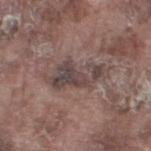illumination: white-light illumination | size: about 6.5 mm | subject: male, aged approximately 75 | location: the leg | imaging modality: total-body-photography crop, ~15 mm field of view.